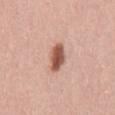Impression: The lesion was tiled from a total-body skin photograph and was not biopsied. Acquisition and patient details: The lesion is located on the mid back. A male patient, aged 48 to 52. A lesion tile, about 15 mm wide, cut from a 3D total-body photograph.